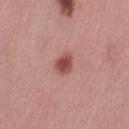{
  "patient": {
    "sex": "female",
    "age_approx": 30
  },
  "site": "leg",
  "image": {
    "source": "total-body photography crop",
    "field_of_view_mm": 15
  }
}